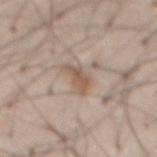Image and clinical context:
A roughly 15 mm field-of-view crop from a total-body skin photograph. About 3.5 mm across. This is a white-light tile. The lesion is on the abdomen. A male subject aged around 55.